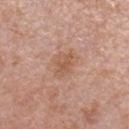Case summary:
* notes · catalogued during a skin exam; not biopsied
* lesion size · ≈3.5 mm
* subject · female, about 40 years old
* automated lesion analysis · a lesion area of about 7 mm², a shape eccentricity near 0.75, and two-axis asymmetry of about 0.25; an average lesion color of about L≈57 a*≈21 b*≈31 (CIELAB), a lesion–skin lightness drop of about 7, and a lesion-to-skin contrast of about 5.5 (normalized; higher = more distinct); a border-irregularity index near 3/10, internal color variation of about 2.5 on a 0–10 scale, and radial color variation of about 1; a detector confidence of about 100 out of 100 that the crop contains a lesion
* tile lighting · white-light illumination
* image · ~15 mm tile from a whole-body skin photo
* location · the right lower leg